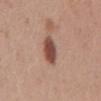Clinical summary: The lesion is located on the mid back. A male patient, aged around 30. Automated image analysis of the tile measured a lesion area of about 7.5 mm² and a shape eccentricity near 0.8. The analysis additionally found border irregularity of about 1.5 on a 0–10 scale, a color-variation rating of about 3/10, and radial color variation of about 1. A roughly 15 mm field-of-view crop from a total-body skin photograph. Approximately 4 mm at its widest.